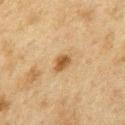No biopsy was performed on this lesion — it was imaged during a full skin examination and was not determined to be concerning.
A 15 mm close-up extracted from a 3D total-body photography capture.
From the chest.
The subject is a male aged approximately 75.